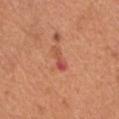Case summary:
– notes — total-body-photography surveillance lesion; no biopsy
– site — the chest
– image — 15 mm crop, total-body photography
– subject — male, aged around 65
– automated lesion analysis — an area of roughly 3 mm², a shape eccentricity near 0.95, and a shape-asymmetry score of about 0.35 (0 = symmetric); a classifier nevus-likeness of about 0/100
– lesion size — ~3 mm (longest diameter)
– tile lighting — white-light illumination